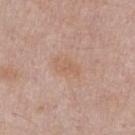This lesion was catalogued during total-body skin photography and was not selected for biopsy.
A male subject approximately 55 years of age.
Captured under white-light illumination.
Automated image analysis of the tile measured a border-irregularity rating of about 5/10, internal color variation of about 0.5 on a 0–10 scale, and peripheral color asymmetry of about 0.5. It also reported an automated nevus-likeness rating near 0 out of 100 and lesion-presence confidence of about 100/100.
On the chest.
This image is a 15 mm lesion crop taken from a total-body photograph.